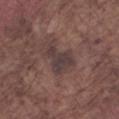This lesion was catalogued during total-body skin photography and was not selected for biopsy.
A male subject, about 75 years old.
Located on the left forearm.
Automated image analysis of the tile measured a border-irregularity index near 5.5/10 and a peripheral color-asymmetry measure near 0.5. The analysis additionally found a nevus-likeness score of about 0/100 and a detector confidence of about 90 out of 100 that the crop contains a lesion.
The recorded lesion diameter is about 4 mm.
A region of skin cropped from a whole-body photographic capture, roughly 15 mm wide.